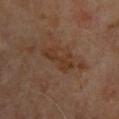notes: total-body-photography surveillance lesion; no biopsy
lighting: cross-polarized
TBP lesion metrics: a shape eccentricity near 0.9; a lesion color around L≈33 a*≈18 b*≈27 in CIELAB and a lesion-to-skin contrast of about 6.5 (normalized; higher = more distinct)
patient: male, aged 63 to 67
body site: the upper back
image: ~15 mm crop, total-body skin-cancer survey
lesion size: ≈4 mm